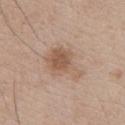Q: Was this lesion biopsied?
A: imaged on a skin check; not biopsied
Q: How was this image acquired?
A: 15 mm crop, total-body photography
Q: How was the tile lit?
A: white-light illumination
Q: What are the patient's age and sex?
A: male, about 65 years old
Q: Automated lesion metrics?
A: a lesion color around L≈56 a*≈17 b*≈29 in CIELAB, a lesion–skin lightness drop of about 9, and a normalized lesion–skin contrast near 6.5; border irregularity of about 4 on a 0–10 scale, a color-variation rating of about 4/10, and a peripheral color-asymmetry measure near 1.5; a nevus-likeness score of about 55/100 and a lesion-detection confidence of about 100/100
Q: What is the anatomic site?
A: the chest
Q: How large is the lesion?
A: about 5 mm